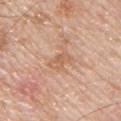Clinical impression: No biopsy was performed on this lesion — it was imaged during a full skin examination and was not determined to be concerning. Acquisition and patient details: A roughly 15 mm field-of-view crop from a total-body skin photograph. A male subject, in their 60s. The lesion's longest dimension is about 3 mm. An algorithmic analysis of the crop reported an area of roughly 4.5 mm², an eccentricity of roughly 0.75, and a shape-asymmetry score of about 0.3 (0 = symmetric). And it measured an average lesion color of about L≈61 a*≈21 b*≈33 (CIELAB), about 7 CIELAB-L* units darker than the surrounding skin, and a lesion-to-skin contrast of about 5 (normalized; higher = more distinct). The software also gave a border-irregularity rating of about 3.5/10, a within-lesion color-variation index near 2/10, and radial color variation of about 0.5. On the chest. Captured under white-light illumination.